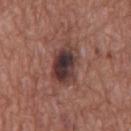biopsy status = no biopsy performed (imaged during a skin exam)
patient = male, approximately 75 years of age
image = ~15 mm tile from a whole-body skin photo
site = the chest